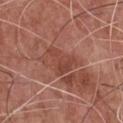This lesion was catalogued during total-body skin photography and was not selected for biopsy. A male patient approximately 75 years of age. The lesion is on the chest. A roughly 15 mm field-of-view crop from a total-body skin photograph.